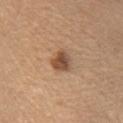Imaged during a routine full-body skin examination; the lesion was not biopsied and no histopathology is available. A female patient aged 58 to 62. Located on the chest. A lesion tile, about 15 mm wide, cut from a 3D total-body photograph. Approximately 3 mm at its widest.